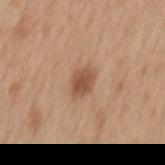Assessment:
Captured during whole-body skin photography for melanoma surveillance; the lesion was not biopsied.